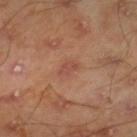workup — total-body-photography surveillance lesion; no biopsy | automated lesion analysis — a lesion area of about 2.5 mm² and a symmetry-axis asymmetry near 0.3; an automated nevus-likeness rating near 10 out of 100 | subject — male, in their mid- to late 40s | size — about 2.5 mm | illumination — cross-polarized illumination | image source — ~15 mm crop, total-body skin-cancer survey | site — the right lower leg.